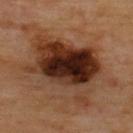notes = no biopsy performed (imaged during a skin exam)
size = ≈10 mm
tile lighting = cross-polarized illumination
image source = 15 mm crop, total-body photography
subject = in their mid-60s
site = the upper back